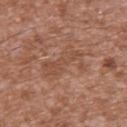Clinical summary:
The lesion's longest dimension is about 6 mm. Located on the back. A roughly 15 mm field-of-view crop from a total-body skin photograph. Imaged with white-light lighting. The patient is a male about 45 years old.